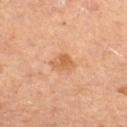The lesion was photographed on a routine skin check and not biopsied; there is no pathology result.
The tile uses cross-polarized illumination.
The lesion is on the right thigh.
Approximately 2.5 mm at its widest.
Cropped from a total-body skin-imaging series; the visible field is about 15 mm.
A female subject, in their mid-40s.
The total-body-photography lesion software estimated a lesion area of about 5 mm² and an outline eccentricity of about 0.4 (0 = round, 1 = elongated). And it measured an average lesion color of about L≈60 a*≈24 b*≈38 (CIELAB) and a lesion–skin lightness drop of about 9. It also reported a border-irregularity rating of about 2.5/10, internal color variation of about 3.5 on a 0–10 scale, and peripheral color asymmetry of about 1. The software also gave a nevus-likeness score of about 5/100 and a lesion-detection confidence of about 100/100.